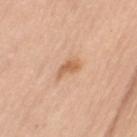Notes:
- notes: catalogued during a skin exam; not biopsied
- diameter: ≈3 mm
- subject: female, about 65 years old
- location: the left upper arm
- illumination: white-light
- acquisition: ~15 mm crop, total-body skin-cancer survey